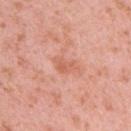Findings:
• notes: no biopsy performed (imaged during a skin exam)
• TBP lesion metrics: a nevus-likeness score of about 0/100 and a detector confidence of about 100 out of 100 that the crop contains a lesion
• patient: female, aged 38 to 42
• image source: ~15 mm tile from a whole-body skin photo
• body site: the left upper arm
• lesion diameter: about 3.5 mm
• tile lighting: white-light